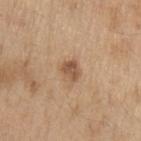notes=imaged on a skin check; not biopsied
image source=15 mm crop, total-body photography
site=the left upper arm
patient=male, aged 68 to 72
lighting=white-light illumination
lesion diameter=about 2.5 mm
automated metrics=an area of roughly 4 mm² and an eccentricity of roughly 0.6; an automated nevus-likeness rating near 40 out of 100 and a detector confidence of about 100 out of 100 that the crop contains a lesion